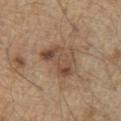biopsy status = total-body-photography surveillance lesion; no biopsy | subject = male, aged around 70 | imaging modality = ~15 mm tile from a whole-body skin photo | diameter = ~5 mm (longest diameter) | anatomic site = the chest.